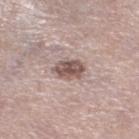Q: Was a biopsy performed?
A: no biopsy performed (imaged during a skin exam)
Q: Patient demographics?
A: female, aged approximately 65
Q: What is the imaging modality?
A: ~15 mm crop, total-body skin-cancer survey
Q: Lesion location?
A: the left lower leg